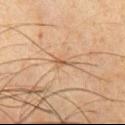A male subject, aged approximately 60.
Cropped from a total-body skin-imaging series; the visible field is about 15 mm.
The lesion is located on the front of the torso.
The lesion's longest dimension is about 2.5 mm.